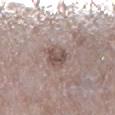Findings:
* notes — imaged on a skin check; not biopsied
* image-analysis metrics — a lesion area of about 5 mm² and an outline eccentricity of about 0.55 (0 = round, 1 = elongated); roughly 10 lightness units darker than nearby skin; an automated nevus-likeness rating near 0 out of 100 and a lesion-detection confidence of about 100/100
* anatomic site — the right lower leg
* imaging modality — ~15 mm crop, total-body skin-cancer survey
* patient — male, aged approximately 65
* lesion size — ~3 mm (longest diameter)